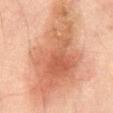Part of a total-body skin-imaging series; this lesion was reviewed on a skin check and was not flagged for biopsy.
On the mid back.
The patient is a male in their mid-60s.
The total-body-photography lesion software estimated an average lesion color of about L≈49 a*≈20 b*≈29 (CIELAB) and roughly 10 lightness units darker than nearby skin.
Cropped from a whole-body photographic skin survey; the tile spans about 15 mm.
Longest diameter approximately 13 mm.
Captured under cross-polarized illumination.